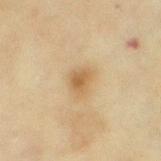| feature | finding |
|---|---|
| notes | total-body-photography surveillance lesion; no biopsy |
| automated metrics | an average lesion color of about L≈53 a*≈14 b*≈35 (CIELAB), a lesion–skin lightness drop of about 9, and a lesion-to-skin contrast of about 7 (normalized; higher = more distinct); border irregularity of about 2.5 on a 0–10 scale, internal color variation of about 2.5 on a 0–10 scale, and peripheral color asymmetry of about 1; a classifier nevus-likeness of about 65/100 and a lesion-detection confidence of about 100/100 |
| diameter | about 3 mm |
| body site | the left thigh |
| patient | female, roughly 55 years of age |
| image | ~15 mm tile from a whole-body skin photo |